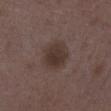biopsy status: total-body-photography surveillance lesion; no biopsy | lighting: white-light illumination | lesion diameter: ≈4 mm | automated metrics: an area of roughly 9 mm², an eccentricity of roughly 0.7, and two-axis asymmetry of about 0.2; a mean CIELAB color near L≈33 a*≈13 b*≈20, a lesion–skin lightness drop of about 8, and a lesion-to-skin contrast of about 8.5 (normalized; higher = more distinct); a border-irregularity index near 2/10, internal color variation of about 2.5 on a 0–10 scale, and a peripheral color-asymmetry measure near 1 | image: ~15 mm crop, total-body skin-cancer survey | site: the leg | patient: female, in their mid- to late 30s.